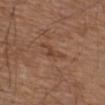Case summary:
• follow-up — total-body-photography surveillance lesion; no biopsy
• image — total-body-photography crop, ~15 mm field of view
• body site — the upper back
• diameter — ≈4 mm
• subject — male, in their mid-70s
• tile lighting — white-light